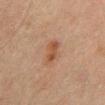<tbp_lesion>
<biopsy_status>not biopsied; imaged during a skin examination</biopsy_status>
<lighting>cross-polarized</lighting>
<image>
  <source>total-body photography crop</source>
  <field_of_view_mm>15</field_of_view_mm>
</image>
<lesion_size>
  <long_diameter_mm_approx>3.0</long_diameter_mm_approx>
</lesion_size>
<patient>
  <sex>male</sex>
  <age_approx>65</age_approx>
</patient>
<site>back</site>
</tbp_lesion>